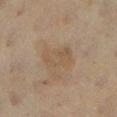Q: Was a biopsy performed?
A: imaged on a skin check; not biopsied
Q: Where on the body is the lesion?
A: the left lower leg
Q: Illumination type?
A: cross-polarized
Q: What are the patient's age and sex?
A: female, in their mid-50s
Q: How was this image acquired?
A: ~15 mm crop, total-body skin-cancer survey
Q: What did automated image analysis measure?
A: a lesion color around L≈45 a*≈12 b*≈27 in CIELAB and about 5 CIELAB-L* units darker than the surrounding skin; a border-irregularity index near 6/10, internal color variation of about 2 on a 0–10 scale, and a peripheral color-asymmetry measure near 0.5; an automated nevus-likeness rating near 0 out of 100 and a lesion-detection confidence of about 100/100
Q: How large is the lesion?
A: about 4 mm